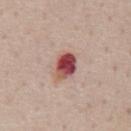This lesion was catalogued during total-body skin photography and was not selected for biopsy.
An algorithmic analysis of the crop reported a lesion–skin lightness drop of about 19 and a normalized lesion–skin contrast near 12.5. The analysis additionally found a nevus-likeness score of about 0/100 and lesion-presence confidence of about 100/100.
A close-up tile cropped from a whole-body skin photograph, about 15 mm across.
A male patient roughly 60 years of age.
Located on the abdomen.